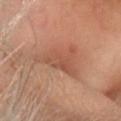Notes:
* follow-up — no biopsy performed (imaged during a skin exam)
* anatomic site — the head or neck
* image — 15 mm crop, total-body photography
* lighting — cross-polarized illumination
* lesion diameter — ≈6 mm
* subject — female, about 60 years old